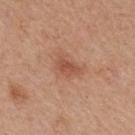Captured during whole-body skin photography for melanoma surveillance; the lesion was not biopsied. A male patient, aged approximately 65. The tile uses white-light illumination. About 3 mm across. Cropped from a whole-body photographic skin survey; the tile spans about 15 mm. Automated image analysis of the tile measured an average lesion color of about L≈52 a*≈25 b*≈32 (CIELAB), roughly 9 lightness units darker than nearby skin, and a normalized border contrast of about 6.5. The analysis additionally found a border-irregularity rating of about 2.5/10, internal color variation of about 2.5 on a 0–10 scale, and a peripheral color-asymmetry measure near 1. The software also gave an automated nevus-likeness rating near 55 out of 100 and a lesion-detection confidence of about 100/100. From the upper back.